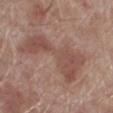Captured during whole-body skin photography for melanoma surveillance; the lesion was not biopsied. A close-up tile cropped from a whole-body skin photograph, about 15 mm across. On the left lower leg. The subject is a male aged 68 to 72.